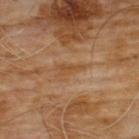follow-up = imaged on a skin check; not biopsied | image source = 15 mm crop, total-body photography | TBP lesion metrics = a footprint of about 3 mm²; a border-irregularity index near 5/10, a color-variation rating of about 0.5/10, and a peripheral color-asymmetry measure near 0; a classifier nevus-likeness of about 0/100 | body site = the front of the torso | patient = male, aged 58–62 | diameter = about 3 mm.